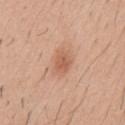Impression: Part of a total-body skin-imaging series; this lesion was reviewed on a skin check and was not flagged for biopsy. Image and clinical context: Captured under white-light illumination. A 15 mm close-up tile from a total-body photography series done for melanoma screening. From the mid back. The patient is a male aged around 40. An algorithmic analysis of the crop reported a footprint of about 4.5 mm², a shape eccentricity near 0.65, and a shape-asymmetry score of about 0.15 (0 = symmetric). And it measured an average lesion color of about L≈59 a*≈24 b*≈32 (CIELAB) and about 9 CIELAB-L* units darker than the surrounding skin. It also reported a classifier nevus-likeness of about 90/100 and lesion-presence confidence of about 100/100. Approximately 2.5 mm at its widest.